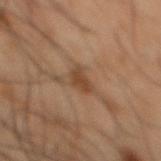| feature | finding |
|---|---|
| follow-up | total-body-photography surveillance lesion; no biopsy |
| anatomic site | the mid back |
| subject | male, aged around 50 |
| acquisition | 15 mm crop, total-body photography |